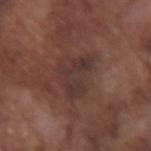Case summary:
– follow-up — total-body-photography surveillance lesion; no biopsy
– body site — the right forearm
– acquisition — total-body-photography crop, ~15 mm field of view
– illumination — white-light illumination
– subject — male, aged approximately 75